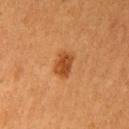notes: catalogued during a skin exam; not biopsied | tile lighting: cross-polarized | anatomic site: the left upper arm | acquisition: ~15 mm crop, total-body skin-cancer survey | subject: female, aged approximately 55 | lesion size: ≈3.5 mm | automated lesion analysis: a mean CIELAB color near L≈41 a*≈24 b*≈37, roughly 10 lightness units darker than nearby skin, and a normalized lesion–skin contrast near 8.5; a border-irregularity rating of about 2.5/10, a within-lesion color-variation index near 2.5/10, and a peripheral color-asymmetry measure near 1; an automated nevus-likeness rating near 95 out of 100 and lesion-presence confidence of about 100/100.